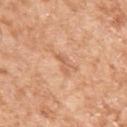The lesion was photographed on a routine skin check and not biopsied; there is no pathology result.
A female patient, aged approximately 75.
A close-up tile cropped from a whole-body skin photograph, about 15 mm across.
The lesion is located on the left upper arm.